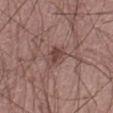This lesion was catalogued during total-body skin photography and was not selected for biopsy.
A roughly 15 mm field-of-view crop from a total-body skin photograph.
A male subject, aged 58 to 62.
The tile uses white-light illumination.
Longest diameter approximately 3 mm.
Located on the abdomen.